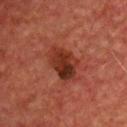{
  "biopsy_status": "not biopsied; imaged during a skin examination",
  "patient": {
    "sex": "male",
    "age_approx": 65
  },
  "lesion_size": {
    "long_diameter_mm_approx": 4.5
  },
  "lighting": "cross-polarized",
  "image": {
    "source": "total-body photography crop",
    "field_of_view_mm": 15
  },
  "automated_metrics": {
    "border_irregularity_0_10": 2.5,
    "color_variation_0_10": 7.0,
    "peripheral_color_asymmetry": 2.5,
    "nevus_likeness_0_100": 50,
    "lesion_detection_confidence_0_100": 100
  },
  "site": "chest"
}